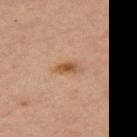follow-up — total-body-photography surveillance lesion; no biopsy | image source — ~15 mm tile from a whole-body skin photo | subject — male, approximately 30 years of age | location — the left upper arm.